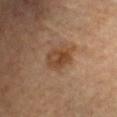Part of a total-body skin-imaging series; this lesion was reviewed on a skin check and was not flagged for biopsy.
Automated tile analysis of the lesion measured a lesion–skin lightness drop of about 9 and a normalized border contrast of about 7.5.
Imaged with cross-polarized lighting.
A female patient, roughly 60 years of age.
Cropped from a whole-body photographic skin survey; the tile spans about 15 mm.
Approximately 3.5 mm at its widest.
From the chest.